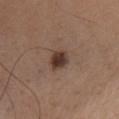| feature | finding |
|---|---|
| workup | no biopsy performed (imaged during a skin exam) |
| lesion diameter | ≈2.5 mm |
| acquisition | 15 mm crop, total-body photography |
| tile lighting | white-light |
| body site | the chest |
| subject | female, aged 43–47 |